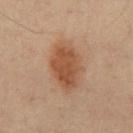follow-up — imaged on a skin check; not biopsied
acquisition — 15 mm crop, total-body photography
patient — male, aged approximately 55
anatomic site — the front of the torso